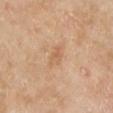Clinical impression: Imaged during a routine full-body skin examination; the lesion was not biopsied and no histopathology is available. Image and clinical context: Imaged with cross-polarized lighting. An algorithmic analysis of the crop reported a shape eccentricity near 0.85 and two-axis asymmetry of about 0.35. And it measured an average lesion color of about L≈61 a*≈20 b*≈35 (CIELAB), a lesion–skin lightness drop of about 7, and a normalized lesion–skin contrast near 5. It also reported a border-irregularity index near 3.5/10, a color-variation rating of about 2/10, and a peripheral color-asymmetry measure near 0.5. The lesion's longest dimension is about 3 mm. A male subject aged around 65. The lesion is on the left lower leg. A 15 mm close-up extracted from a 3D total-body photography capture.